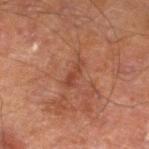Impression:
The lesion was tiled from a total-body skin photograph and was not biopsied.
Image and clinical context:
A male patient in their 70s. Located on the left lower leg. A 15 mm close-up tile from a total-body photography series done for melanoma screening. Captured under cross-polarized illumination.